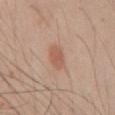Image and clinical context:
A close-up tile cropped from a whole-body skin photograph, about 15 mm across. The recorded lesion diameter is about 3 mm. The lesion is located on the lower back. Imaged with white-light lighting. A male subject, aged around 25.